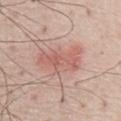Notes:
* diameter — about 6 mm
* image source — total-body-photography crop, ~15 mm field of view
* patient — male, aged approximately 70
* location — the chest